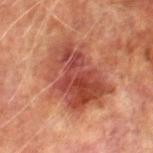Impression:
This lesion was catalogued during total-body skin photography and was not selected for biopsy.
Clinical summary:
A male subject, in their mid-70s. A close-up tile cropped from a whole-body skin photograph, about 15 mm across. Located on the right thigh. The lesion-visualizer software estimated an automated nevus-likeness rating near 15 out of 100 and a lesion-detection confidence of about 100/100.